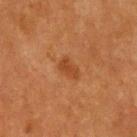{"biopsy_status": "not biopsied; imaged during a skin examination", "patient": {"sex": "male", "age_approx": 75}, "lighting": "cross-polarized", "site": "upper back", "image": {"source": "total-body photography crop", "field_of_view_mm": 15}}